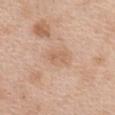follow-up = no biopsy performed (imaged during a skin exam) | imaging modality = ~15 mm tile from a whole-body skin photo | patient = female, aged 38 to 42 | diameter = about 2.5 mm | site = the front of the torso.